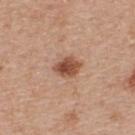{
  "biopsy_status": "not biopsied; imaged during a skin examination",
  "lesion_size": {
    "long_diameter_mm_approx": 3.5
  },
  "lighting": "white-light",
  "site": "upper back",
  "patient": {
    "sex": "male",
    "age_approx": 55
  },
  "image": {
    "source": "total-body photography crop",
    "field_of_view_mm": 15
  }
}